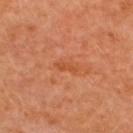Q: Was a biopsy performed?
A: imaged on a skin check; not biopsied
Q: Automated lesion metrics?
A: an outline eccentricity of about 0.95 (0 = round, 1 = elongated) and two-axis asymmetry of about 0.35; a lesion color around L≈51 a*≈30 b*≈40 in CIELAB, roughly 6 lightness units darker than nearby skin, and a lesion-to-skin contrast of about 5.5 (normalized; higher = more distinct)
Q: What is the anatomic site?
A: the upper back
Q: Who is the patient?
A: female, aged 48 to 52
Q: Illumination type?
A: cross-polarized
Q: What kind of image is this?
A: ~15 mm tile from a whole-body skin photo